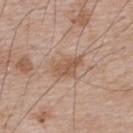<case>
  <biopsy_status>not biopsied; imaged during a skin examination</biopsy_status>
  <patient>
    <sex>male</sex>
    <age_approx>65</age_approx>
  </patient>
  <automated_metrics>
    <area_mm2_approx>8.5</area_mm2_approx>
    <vs_skin_darker_L>8.0</vs_skin_darker_L>
    <border_irregularity_0_10>3.5</border_irregularity_0_10>
    <peripheral_color_asymmetry>1.5</peripheral_color_asymmetry>
    <nevus_likeness_0_100>35</nevus_likeness_0_100>
    <lesion_detection_confidence_0_100>100</lesion_detection_confidence_0_100>
  </automated_metrics>
  <site>upper back</site>
  <lighting>white-light</lighting>
  <image>
    <source>total-body photography crop</source>
    <field_of_view_mm>15</field_of_view_mm>
  </image>
  <lesion_size>
    <long_diameter_mm_approx>4.0</long_diameter_mm_approx>
  </lesion_size>
</case>